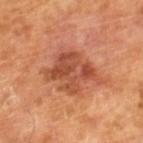Q: Was this lesion biopsied?
A: no biopsy performed (imaged during a skin exam)
Q: What did automated image analysis measure?
A: a border-irregularity index near 4.5/10, a within-lesion color-variation index near 5.5/10, and peripheral color asymmetry of about 2
Q: Patient demographics?
A: male, aged approximately 65
Q: What kind of image is this?
A: ~15 mm tile from a whole-body skin photo
Q: What is the lesion's diameter?
A: ~6 mm (longest diameter)